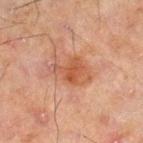Part of a total-body skin-imaging series; this lesion was reviewed on a skin check and was not flagged for biopsy.
Automated image analysis of the tile measured an average lesion color of about L≈45 a*≈21 b*≈29 (CIELAB), a lesion–skin lightness drop of about 8, and a normalized lesion–skin contrast near 6.5. The analysis additionally found a detector confidence of about 100 out of 100 that the crop contains a lesion.
The recorded lesion diameter is about 5 mm.
Captured under cross-polarized illumination.
The lesion is located on the leg.
A 15 mm close-up tile from a total-body photography series done for melanoma screening.
The patient is a male roughly 45 years of age.